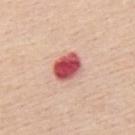acquisition: 15 mm crop, total-body photography | size: ~3.5 mm (longest diameter) | subject: male, aged 63 to 67 | body site: the mid back | lighting: white-light.